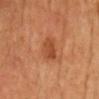Findings:
– workup · total-body-photography surveillance lesion; no biopsy
– anatomic site · the chest
– imaging modality · 15 mm crop, total-body photography
– TBP lesion metrics · a mean CIELAB color near L≈41 a*≈24 b*≈33, about 8 CIELAB-L* units darker than the surrounding skin, and a normalized lesion–skin contrast near 6.5
– patient · male, in their mid- to late 60s
– lighting · cross-polarized illumination